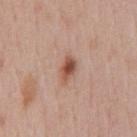Impression:
The lesion was tiled from a total-body skin photograph and was not biopsied.
Image and clinical context:
The lesion's longest dimension is about 3.5 mm. Imaged with white-light lighting. A 15 mm crop from a total-body photograph taken for skin-cancer surveillance. Located on the chest. An algorithmic analysis of the crop reported roughly 13 lightness units darker than nearby skin and a normalized border contrast of about 9. The analysis additionally found a border-irregularity index near 3/10, a within-lesion color-variation index near 4.5/10, and radial color variation of about 1. The software also gave a classifier nevus-likeness of about 90/100 and lesion-presence confidence of about 100/100. The patient is a male aged approximately 55.